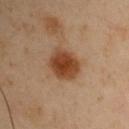{"site": "chest", "patient": {"sex": "male", "age_approx": 55}, "image": {"source": "total-body photography crop", "field_of_view_mm": 15}}